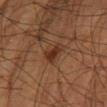biopsy_status: not biopsied; imaged during a skin examination
patient:
  sex: male
  age_approx: 60
image:
  source: total-body photography crop
  field_of_view_mm: 15
lighting: cross-polarized
lesion_size:
  long_diameter_mm_approx: 3.0
site: right lower leg